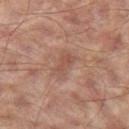Assessment:
The lesion was photographed on a routine skin check and not biopsied; there is no pathology result.
Clinical summary:
A 15 mm crop from a total-body photograph taken for skin-cancer surveillance. Approximately 3.5 mm at its widest. A male subject approximately 65 years of age. On the left thigh.